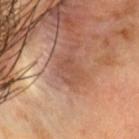Q: Was this lesion biopsied?
A: total-body-photography surveillance lesion; no biopsy
Q: What is the lesion's diameter?
A: ≈3 mm
Q: Patient demographics?
A: male, aged 58–62
Q: What is the anatomic site?
A: the head or neck
Q: How was the tile lit?
A: cross-polarized
Q: What is the imaging modality?
A: 15 mm crop, total-body photography